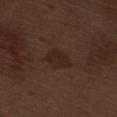<case>
<biopsy_status>not biopsied; imaged during a skin examination</biopsy_status>
<automated_metrics>
  <border_irregularity_0_10>3.0</border_irregularity_0_10>
  <color_variation_0_10>3.0</color_variation_0_10>
  <peripheral_color_asymmetry>1.0</peripheral_color_asymmetry>
</automated_metrics>
<lesion_size>
  <long_diameter_mm_approx>3.5</long_diameter_mm_approx>
</lesion_size>
<image>
  <source>total-body photography crop</source>
  <field_of_view_mm>15</field_of_view_mm>
</image>
<lighting>white-light</lighting>
<patient>
  <sex>male</sex>
  <age_approx>70</age_approx>
</patient>
<site>right thigh</site>
</case>